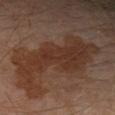follow-up: catalogued during a skin exam; not biopsied
tile lighting: cross-polarized
subject: male, approximately 60 years of age
imaging modality: total-body-photography crop, ~15 mm field of view
size: ≈11 mm
location: the left lower leg